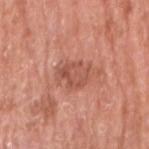Captured during whole-body skin photography for melanoma surveillance; the lesion was not biopsied.
The tile uses white-light illumination.
A lesion tile, about 15 mm wide, cut from a 3D total-body photograph.
A male patient roughly 80 years of age.
The lesion's longest dimension is about 3.5 mm.
An algorithmic analysis of the crop reported an outline eccentricity of about 0.6 (0 = round, 1 = elongated). The analysis additionally found an average lesion color of about L≈53 a*≈27 b*≈30 (CIELAB) and a lesion-to-skin contrast of about 6 (normalized; higher = more distinct). The software also gave a border-irregularity index near 4/10 and a color-variation rating of about 3.5/10. And it measured a detector confidence of about 100 out of 100 that the crop contains a lesion.
Located on the right upper arm.